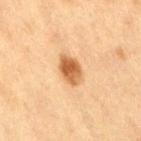Findings:
• workup · catalogued during a skin exam; not biopsied
• patient · female, aged 38–42
• anatomic site · the right thigh
• lesion diameter · ~3.5 mm (longest diameter)
• image · total-body-photography crop, ~15 mm field of view
• lighting · cross-polarized illumination